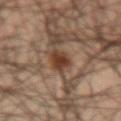{"biopsy_status": "not biopsied; imaged during a skin examination", "site": "arm", "lesion_size": {"long_diameter_mm_approx": 4.0}, "lighting": "cross-polarized", "image": {"source": "total-body photography crop", "field_of_view_mm": 15}, "patient": {"sex": "male", "age_approx": 50}}